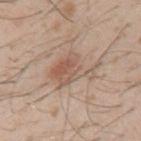Part of a total-body skin-imaging series; this lesion was reviewed on a skin check and was not flagged for biopsy.
The lesion is on the left upper arm.
Captured under white-light illumination.
Automated image analysis of the tile measured a lesion area of about 13 mm², an outline eccentricity of about 0.8 (0 = round, 1 = elongated), and a symmetry-axis asymmetry near 0.45. It also reported a lesion color around L≈57 a*≈17 b*≈27 in CIELAB and a lesion-to-skin contrast of about 6 (normalized; higher = more distinct).
About 6 mm across.
A male subject, aged approximately 30.
A 15 mm close-up extracted from a 3D total-body photography capture.